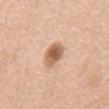Impression: This lesion was catalogued during total-body skin photography and was not selected for biopsy. Acquisition and patient details: The lesion-visualizer software estimated a mean CIELAB color near L≈60 a*≈21 b*≈32. It also reported a border-irregularity rating of about 1.5/10 and peripheral color asymmetry of about 1.5. Located on the chest. A close-up tile cropped from a whole-body skin photograph, about 15 mm across. The tile uses white-light illumination. Longest diameter approximately 3.5 mm. A male subject aged 73–77.